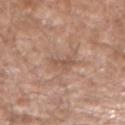biopsy status = catalogued during a skin exam; not biopsied
tile lighting = white-light
subject = male, about 60 years old
anatomic site = the left forearm
imaging modality = 15 mm crop, total-body photography
size = ~2.5 mm (longest diameter)